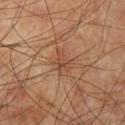Case summary:
– follow-up: imaged on a skin check; not biopsied
– body site: the left upper arm
– acquisition: 15 mm crop, total-body photography
– subject: male, roughly 75 years of age